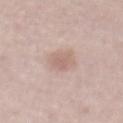  biopsy_status: not biopsied; imaged during a skin examination
  lesion_size:
    long_diameter_mm_approx: 3.0
  automated_metrics:
    cielab_L: 63
    cielab_a: 17
    cielab_b: 24
    vs_skin_darker_L: 8.0
    vs_skin_contrast_norm: 5.5
  site: abdomen
  patient:
    sex: male
    age_approx: 75
  image:
    source: total-body photography crop
    field_of_view_mm: 15
  lighting: white-light